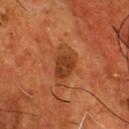Assessment: No biopsy was performed on this lesion — it was imaged during a full skin examination and was not determined to be concerning. Background: A male patient, about 55 years old. This image is a 15 mm lesion crop taken from a total-body photograph. Imaged with cross-polarized lighting. An algorithmic analysis of the crop reported a footprint of about 9.5 mm², an eccentricity of roughly 0.85, and a shape-asymmetry score of about 0.25 (0 = symmetric). The software also gave a mean CIELAB color near L≈38 a*≈25 b*≈35, a lesion–skin lightness drop of about 9, and a normalized border contrast of about 7. The software also gave a nevus-likeness score of about 15/100 and a lesion-detection confidence of about 100/100. The lesion is located on the chest. Measured at roughly 5 mm in maximum diameter.